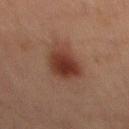Imaged during a routine full-body skin examination; the lesion was not biopsied and no histopathology is available.
A male subject, roughly 65 years of age.
On the abdomen.
The lesion-visualizer software estimated a border-irregularity rating of about 2/10, a color-variation rating of about 4/10, and radial color variation of about 1.
A 15 mm close-up extracted from a 3D total-body photography capture.